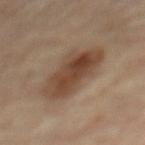The lesion was photographed on a routine skin check and not biopsied; there is no pathology result. Cropped from a whole-body photographic skin survey; the tile spans about 15 mm. The recorded lesion diameter is about 6 mm. From the back. A male patient, about 85 years old or older. The tile uses cross-polarized illumination.